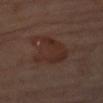Recorded during total-body skin imaging; not selected for excision or biopsy. A female patient aged 63 to 67. On the left upper arm. The lesion-visualizer software estimated roughly 5 lightness units darker than nearby skin and a normalized lesion–skin contrast near 6.5. The analysis additionally found a border-irregularity rating of about 4/10, internal color variation of about 3 on a 0–10 scale, and peripheral color asymmetry of about 1. The analysis additionally found a nevus-likeness score of about 10/100 and lesion-presence confidence of about 100/100. This is a cross-polarized tile. A close-up tile cropped from a whole-body skin photograph, about 15 mm across. The lesion's longest dimension is about 6 mm.